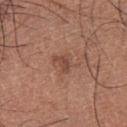Findings:
• biopsy status: catalogued during a skin exam; not biopsied
• imaging modality: ~15 mm crop, total-body skin-cancer survey
• patient: male, in their mid- to late 30s
• anatomic site: the front of the torso
• diameter: ~2.5 mm (longest diameter)
• illumination: white-light illumination
• automated lesion analysis: a symmetry-axis asymmetry near 0.55; a lesion color around L≈45 a*≈22 b*≈27 in CIELAB, about 9 CIELAB-L* units darker than the surrounding skin, and a lesion-to-skin contrast of about 6.5 (normalized; higher = more distinct); a color-variation rating of about 0/10 and radial color variation of about 0; a classifier nevus-likeness of about 5/100 and lesion-presence confidence of about 100/100